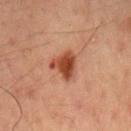The lesion was tiled from a total-body skin photograph and was not biopsied. An algorithmic analysis of the crop reported an area of roughly 7 mm² and a symmetry-axis asymmetry near 0.45. Approximately 3 mm at its widest. The tile uses cross-polarized illumination. From the mid back. This image is a 15 mm lesion crop taken from a total-body photograph. A male subject, aged 48 to 52.